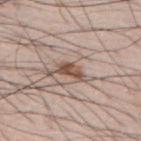  patient:
    sex: male
    age_approx: 75
  image:
    source: total-body photography crop
    field_of_view_mm: 15
  site: upper back